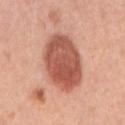Impression:
Recorded during total-body skin imaging; not selected for excision or biopsy.
Acquisition and patient details:
A 15 mm close-up extracted from a 3D total-body photography capture. The lesion is on the right upper arm. A female patient approximately 60 years of age.